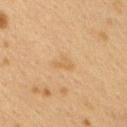diameter: ~3 mm (longest diameter) | acquisition: total-body-photography crop, ~15 mm field of view | subject: female, roughly 40 years of age | lighting: cross-polarized illumination | automated metrics: a lesion color around L≈52 a*≈15 b*≈34 in CIELAB, a lesion–skin lightness drop of about 5, and a lesion-to-skin contrast of about 4.5 (normalized; higher = more distinct) | anatomic site: the right upper arm.